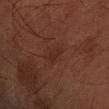Measured at roughly 3 mm in maximum diameter. Captured under cross-polarized illumination. A 15 mm crop from a total-body photograph taken for skin-cancer surveillance. The subject is a male aged approximately 60. On the left forearm.